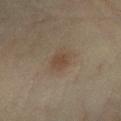Impression:
Imaged during a routine full-body skin examination; the lesion was not biopsied and no histopathology is available.
Background:
The patient is a female in their 60s. A region of skin cropped from a whole-body photographic capture, roughly 15 mm wide. An algorithmic analysis of the crop reported a lesion area of about 4 mm². It also reported a mean CIELAB color near L≈39 a*≈13 b*≈25, about 6 CIELAB-L* units darker than the surrounding skin, and a normalized border contrast of about 6. And it measured internal color variation of about 2 on a 0–10 scale and radial color variation of about 0.5. The tile uses cross-polarized illumination. The lesion's longest dimension is about 2.5 mm. The lesion is on the left lower leg.